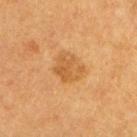patient = female, aged approximately 40
image = 15 mm crop, total-body photography
location = the left lower leg
automated lesion analysis = a within-lesion color-variation index near 3/10 and a peripheral color-asymmetry measure near 1
lesion size = ~3.5 mm (longest diameter)
tile lighting = cross-polarized